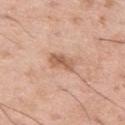Assessment:
Recorded during total-body skin imaging; not selected for excision or biopsy.
Background:
Located on the leg. A male subject approximately 50 years of age. A 15 mm crop from a total-body photograph taken for skin-cancer surveillance.